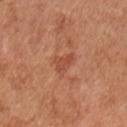A close-up tile cropped from a whole-body skin photograph, about 15 mm across. The subject is a male aged 53–57. Longest diameter approximately 2.5 mm. Imaged with white-light lighting. From the chest.